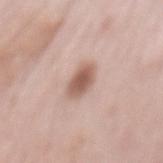<lesion>
<biopsy_status>not biopsied; imaged during a skin examination</biopsy_status>
<lighting>white-light</lighting>
<automated_metrics>
  <color_variation_0_10>3.5</color_variation_0_10>
  <peripheral_color_asymmetry>1.0</peripheral_color_asymmetry>
  <lesion_detection_confidence_0_100>100</lesion_detection_confidence_0_100>
</automated_metrics>
<patient>
  <sex>female</sex>
  <age_approx>65</age_approx>
</patient>
<image>
  <source>total-body photography crop</source>
  <field_of_view_mm>15</field_of_view_mm>
</image>
<site>mid back</site>
<lesion_size>
  <long_diameter_mm_approx>3.5</long_diameter_mm_approx>
</lesion_size>
</lesion>